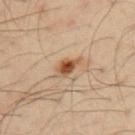{"biopsy_status": "not biopsied; imaged during a skin examination", "site": "left upper arm", "image": {"source": "total-body photography crop", "field_of_view_mm": 15}, "patient": {"sex": "male", "age_approx": 50}}